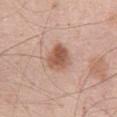biopsy_status: not biopsied; imaged during a skin examination
lesion_size:
  long_diameter_mm_approx: 3.5
site: abdomen
patient:
  sex: male
  age_approx: 50
automated_metrics:
  cielab_L: 56
  cielab_a: 21
  cielab_b: 29
  vs_skin_darker_L: 12.0
  nevus_likeness_0_100: 95
  lesion_detection_confidence_0_100: 100
lighting: white-light
image:
  source: total-body photography crop
  field_of_view_mm: 15